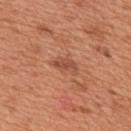This lesion was catalogued during total-body skin photography and was not selected for biopsy. A male patient, approximately 65 years of age. The tile uses white-light illumination. A 15 mm close-up extracted from a 3D total-body photography capture. The total-body-photography lesion software estimated a border-irregularity index near 3.5/10, internal color variation of about 2 on a 0–10 scale, and a peripheral color-asymmetry measure near 0.5. The lesion is located on the upper back. The recorded lesion diameter is about 3 mm.